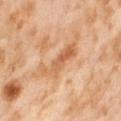Assessment: Imaged during a routine full-body skin examination; the lesion was not biopsied and no histopathology is available.